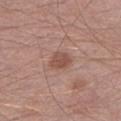Impression:
Captured during whole-body skin photography for melanoma surveillance; the lesion was not biopsied.
Clinical summary:
The lesion is located on the left lower leg. A 15 mm close-up extracted from a 3D total-body photography capture. This is a white-light tile. A male patient aged 53 to 57.